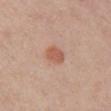Case summary:
• biopsy status — total-body-photography surveillance lesion; no biopsy
• subject — female, aged 23–27
• imaging modality — ~15 mm crop, total-body skin-cancer survey
• body site — the chest